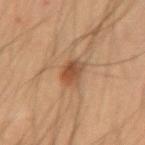| field | value |
|---|---|
| notes | total-body-photography surveillance lesion; no biopsy |
| image | total-body-photography crop, ~15 mm field of view |
| location | the left forearm |
| TBP lesion metrics | a peripheral color-asymmetry measure near 1.5 |
| illumination | cross-polarized |
| subject | male, about 35 years old |